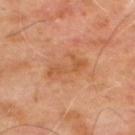Clinical impression: The lesion was tiled from a total-body skin photograph and was not biopsied. Background: Measured at roughly 4.5 mm in maximum diameter. The subject is a male in their mid- to late 60s. A 15 mm crop from a total-body photograph taken for skin-cancer surveillance. The tile uses cross-polarized illumination. The lesion is located on the upper back. The total-body-photography lesion software estimated an area of roughly 7 mm² and a shape eccentricity near 0.9. It also reported a mean CIELAB color near L≈53 a*≈24 b*≈38, roughly 7 lightness units darker than nearby skin, and a lesion-to-skin contrast of about 5.5 (normalized; higher = more distinct). And it measured a classifier nevus-likeness of about 0/100.